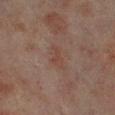Findings:
– image — ~15 mm tile from a whole-body skin photo
– location — the left lower leg
– subject — female, aged approximately 80